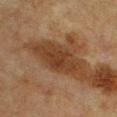Part of a total-body skin-imaging series; this lesion was reviewed on a skin check and was not flagged for biopsy.
The tile uses cross-polarized illumination.
The patient is a male aged around 75.
Measured at roughly 8 mm in maximum diameter.
Cropped from a whole-body photographic skin survey; the tile spans about 15 mm.
Located on the front of the torso.
The lesion-visualizer software estimated a lesion color around L≈31 a*≈17 b*≈27 in CIELAB, roughly 8 lightness units darker than nearby skin, and a normalized border contrast of about 8.5. The software also gave a border-irregularity index near 3/10 and a color-variation rating of about 3/10.